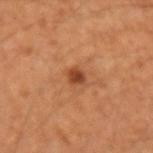The lesion was tiled from a total-body skin photograph and was not biopsied.
On the arm.
About 2 mm across.
Cropped from a whole-body photographic skin survey; the tile spans about 15 mm.
A male subject aged approximately 50.